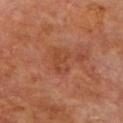{
  "biopsy_status": "not biopsied; imaged during a skin examination",
  "patient": {
    "sex": "male",
    "age_approx": 70
  },
  "image": {
    "source": "total-body photography crop",
    "field_of_view_mm": 15
  },
  "site": "chest"
}